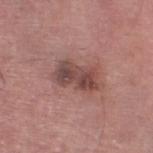Clinical impression: Imaged during a routine full-body skin examination; the lesion was not biopsied and no histopathology is available. Background: A male patient, in their mid- to late 70s. Cropped from a whole-body photographic skin survey; the tile spans about 15 mm. The lesion is located on the left lower leg.